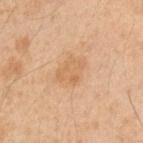<case>
<biopsy_status>not biopsied; imaged during a skin examination</biopsy_status>
<patient>
  <sex>male</sex>
  <age_approx>50</age_approx>
</patient>
<site>arm</site>
<lesion_size>
  <long_diameter_mm_approx>4.0</long_diameter_mm_approx>
</lesion_size>
<automated_metrics>
  <eccentricity>0.6</eccentricity>
  <border_irregularity_0_10>2.5</border_irregularity_0_10>
  <color_variation_0_10>2.0</color_variation_0_10>
  <peripheral_color_asymmetry>0.5</peripheral_color_asymmetry>
  <nevus_likeness_0_100>0</nevus_likeness_0_100>
  <lesion_detection_confidence_0_100>100</lesion_detection_confidence_0_100>
</automated_metrics>
<image>
  <source>total-body photography crop</source>
  <field_of_view_mm>15</field_of_view_mm>
</image>
<lighting>cross-polarized</lighting>
</case>